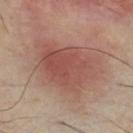Case summary:
- workup: catalogued during a skin exam; not biopsied
- lighting: cross-polarized
- patient: male, aged 33–37
- lesion diameter: ≈8 mm
- acquisition: 15 mm crop, total-body photography
- anatomic site: the front of the torso